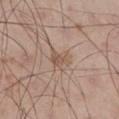Clinical impression:
No biopsy was performed on this lesion — it was imaged during a full skin examination and was not determined to be concerning.